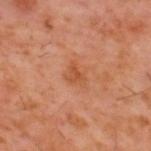Clinical impression: Recorded during total-body skin imaging; not selected for excision or biopsy. Background: The subject is a male roughly 60 years of age. Located on the upper back. A region of skin cropped from a whole-body photographic capture, roughly 15 mm wide. This is a cross-polarized tile.